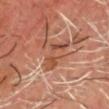Q: Is there a histopathology result?
A: no biopsy performed (imaged during a skin exam)
Q: What kind of image is this?
A: 15 mm crop, total-body photography
Q: What did automated image analysis measure?
A: an area of roughly 6.5 mm², a shape eccentricity near 0.85, and a shape-asymmetry score of about 0.5 (0 = symmetric); border irregularity of about 7 on a 0–10 scale, a within-lesion color-variation index near 2.5/10, and a peripheral color-asymmetry measure near 1; a classifier nevus-likeness of about 0/100 and a detector confidence of about 95 out of 100 that the crop contains a lesion
Q: What is the anatomic site?
A: the head or neck
Q: What is the lesion's diameter?
A: ≈4 mm
Q: Patient demographics?
A: male, aged approximately 70